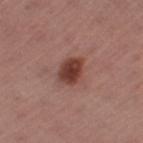Case summary:
• workup — catalogued during a skin exam; not biopsied
• acquisition — 15 mm crop, total-body photography
• tile lighting — white-light illumination
• location — the left thigh
• diameter — ≈3.5 mm
• subject — female, aged approximately 55